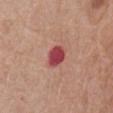Q: Was a biopsy performed?
A: imaged on a skin check; not biopsied
Q: Who is the patient?
A: female, in their mid-70s
Q: What is the anatomic site?
A: the abdomen
Q: What is the imaging modality?
A: ~15 mm crop, total-body skin-cancer survey
Q: What is the lesion's diameter?
A: ~3 mm (longest diameter)
Q: What lighting was used for the tile?
A: white-light